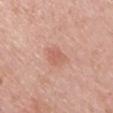Recorded during total-body skin imaging; not selected for excision or biopsy. The patient is a male in their mid- to late 20s. Imaged with white-light lighting. The lesion is located on the chest. A region of skin cropped from a whole-body photographic capture, roughly 15 mm wide.